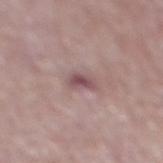biopsy_status: not biopsied; imaged during a skin examination
image:
  source: total-body photography crop
  field_of_view_mm: 15
patient:
  sex: male
  age_approx: 65
site: back